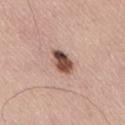patient: male, aged 53 to 57; location: the right thigh; image: total-body-photography crop, ~15 mm field of view; TBP lesion metrics: a within-lesion color-variation index near 8/10.